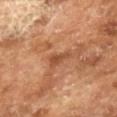subject — male, approximately 65 years of age | lighting — cross-polarized | size — ~2.5 mm (longest diameter) | image source — ~15 mm tile from a whole-body skin photo | automated lesion analysis — an eccentricity of roughly 0.8 and two-axis asymmetry of about 0.5; a border-irregularity index near 5/10, a within-lesion color-variation index near 1/10, and peripheral color asymmetry of about 0.5; a nevus-likeness score of about 0/100 and a detector confidence of about 100 out of 100 that the crop contains a lesion.